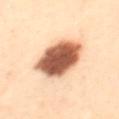Notes:
– biopsy status · total-body-photography surveillance lesion; no biopsy
– lesion diameter · ~6.5 mm (longest diameter)
– site · the mid back
– imaging modality · 15 mm crop, total-body photography
– TBP lesion metrics · roughly 21 lightness units darker than nearby skin
– patient · female, in their 30s